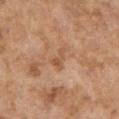Part of a total-body skin-imaging series; this lesion was reviewed on a skin check and was not flagged for biopsy.
Measured at roughly 3 mm in maximum diameter.
Captured under cross-polarized illumination.
An algorithmic analysis of the crop reported a shape eccentricity near 0.9. The analysis additionally found a lesion color around L≈46 a*≈19 b*≈31 in CIELAB, about 7 CIELAB-L* units darker than the surrounding skin, and a normalized border contrast of about 6. The software also gave a border-irregularity index near 7.5/10, a color-variation rating of about 0.5/10, and peripheral color asymmetry of about 0.
A female subject, roughly 60 years of age.
On the left lower leg.
A roughly 15 mm field-of-view crop from a total-body skin photograph.